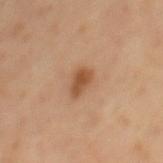<lesion>
  <biopsy_status>not biopsied; imaged during a skin examination</biopsy_status>
  <lesion_size>
    <long_diameter_mm_approx>3.0</long_diameter_mm_approx>
  </lesion_size>
  <site>back</site>
  <lighting>cross-polarized</lighting>
  <patient>
    <sex>male</sex>
    <age_approx>60</age_approx>
  </patient>
  <image>
    <source>total-body photography crop</source>
    <field_of_view_mm>15</field_of_view_mm>
  </image>
</lesion>